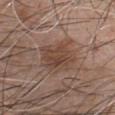Clinical summary:
A male patient in their mid-40s. From the chest. A lesion tile, about 15 mm wide, cut from a 3D total-body photograph.